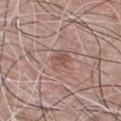- notes — total-body-photography surveillance lesion; no biopsy
- size — about 3 mm
- location — the front of the torso
- patient — male, about 75 years old
- image source — total-body-photography crop, ~15 mm field of view
- automated metrics — a lesion area of about 5 mm², an outline eccentricity of about 0.65 (0 = round, 1 = elongated), and a symmetry-axis asymmetry near 0.25; a mean CIELAB color near L≈53 a*≈19 b*≈25; a border-irregularity rating of about 2.5/10, internal color variation of about 3.5 on a 0–10 scale, and radial color variation of about 1.5; a nevus-likeness score of about 0/100 and lesion-presence confidence of about 100/100